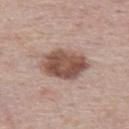Q: What are the patient's age and sex?
A: male, aged 38 to 42
Q: How large is the lesion?
A: ~5.5 mm (longest diameter)
Q: What kind of image is this?
A: ~15 mm tile from a whole-body skin photo
Q: What lighting was used for the tile?
A: white-light illumination
Q: Where on the body is the lesion?
A: the back